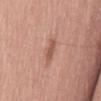* follow-up · imaged on a skin check; not biopsied
* location · the abdomen
* patient · male, approximately 60 years of age
* acquisition · ~15 mm crop, total-body skin-cancer survey